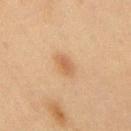Clinical impression:
The lesion was photographed on a routine skin check and not biopsied; there is no pathology result.
Acquisition and patient details:
Approximately 3 mm at its widest. Located on the upper back. A roughly 15 mm field-of-view crop from a total-body skin photograph. A male patient in their mid-40s. Captured under cross-polarized illumination.